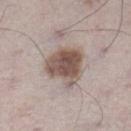Assessment: Captured during whole-body skin photography for melanoma surveillance; the lesion was not biopsied. Acquisition and patient details: Captured under white-light illumination. From the left lower leg. The subject is a male in their 70s. Approximately 5 mm at its widest. A region of skin cropped from a whole-body photographic capture, roughly 15 mm wide. Automated image analysis of the tile measured border irregularity of about 3 on a 0–10 scale, a color-variation rating of about 5/10, and peripheral color asymmetry of about 1.5. And it measured a classifier nevus-likeness of about 75/100 and a lesion-detection confidence of about 100/100.